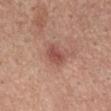Assessment: The lesion was tiled from a total-body skin photograph and was not biopsied. Context: Located on the mid back. The tile uses white-light illumination. This image is a 15 mm lesion crop taken from a total-body photograph. The total-body-photography lesion software estimated a lesion area of about 5 mm², a shape eccentricity near 0.5, and two-axis asymmetry of about 0.2. A male patient, aged 53 to 57.